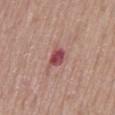Q: Was this lesion biopsied?
A: imaged on a skin check; not biopsied
Q: What are the patient's age and sex?
A: male, in their mid-50s
Q: How large is the lesion?
A: ~2.5 mm (longest diameter)
Q: What did automated image analysis measure?
A: an average lesion color of about L≈48 a*≈30 b*≈21 (CIELAB) and a lesion-to-skin contrast of about 9.5 (normalized; higher = more distinct); border irregularity of about 2.5 on a 0–10 scale, internal color variation of about 4 on a 0–10 scale, and peripheral color asymmetry of about 1; lesion-presence confidence of about 100/100
Q: What is the imaging modality?
A: total-body-photography crop, ~15 mm field of view